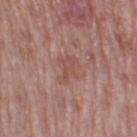Captured during whole-body skin photography for melanoma surveillance; the lesion was not biopsied.
Automated image analysis of the tile measured roughly 6 lightness units darker than nearby skin and a lesion-to-skin contrast of about 5 (normalized; higher = more distinct). And it measured peripheral color asymmetry of about 0.5. The software also gave a nevus-likeness score of about 0/100 and a lesion-detection confidence of about 100/100.
Measured at roughly 3 mm in maximum diameter.
From the left thigh.
Cropped from a total-body skin-imaging series; the visible field is about 15 mm.
A female patient, roughly 60 years of age.